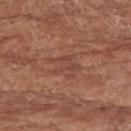Q: Is there a histopathology result?
A: imaged on a skin check; not biopsied
Q: How was this image acquired?
A: ~15 mm tile from a whole-body skin photo
Q: What are the patient's age and sex?
A: female, about 75 years old
Q: What is the anatomic site?
A: the left forearm
Q: What is the lesion's diameter?
A: ~3.5 mm (longest diameter)
Q: What lighting was used for the tile?
A: white-light illumination
Q: Automated lesion metrics?
A: an eccentricity of roughly 0.65 and two-axis asymmetry of about 0.5; a lesion color around L≈45 a*≈24 b*≈27 in CIELAB, roughly 6 lightness units darker than nearby skin, and a lesion-to-skin contrast of about 5 (normalized; higher = more distinct); a border-irregularity index near 7.5/10 and internal color variation of about 2 on a 0–10 scale; an automated nevus-likeness rating near 0 out of 100 and a detector confidence of about 95 out of 100 that the crop contains a lesion